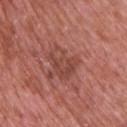Assessment:
The lesion was tiled from a total-body skin photograph and was not biopsied.
Clinical summary:
The subject is a male aged 68 to 72. Longest diameter approximately 4 mm. The lesion is located on the upper back. An algorithmic analysis of the crop reported a mean CIELAB color near L≈45 a*≈26 b*≈27. And it measured a classifier nevus-likeness of about 0/100. A 15 mm close-up tile from a total-body photography series done for melanoma screening.